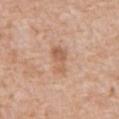The lesion was photographed on a routine skin check and not biopsied; there is no pathology result. A region of skin cropped from a whole-body photographic capture, roughly 15 mm wide. The subject is a male about 60 years old. The lesion is on the back. This is a white-light tile. The recorded lesion diameter is about 3.5 mm.